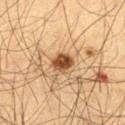Assessment:
No biopsy was performed on this lesion — it was imaged during a full skin examination and was not determined to be concerning.
Image and clinical context:
The lesion-visualizer software estimated a detector confidence of about 100 out of 100 that the crop contains a lesion. From the left thigh. Imaged with cross-polarized lighting. The subject is a male aged 63–67. Longest diameter approximately 3 mm. Cropped from a total-body skin-imaging series; the visible field is about 15 mm.